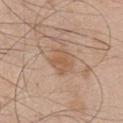Case summary:
* follow-up · no biopsy performed (imaged during a skin exam)
* patient · male, aged 43–47
* automated lesion analysis · a footprint of about 6.5 mm² and an eccentricity of roughly 0.6; a lesion color around L≈57 a*≈19 b*≈32 in CIELAB, a lesion–skin lightness drop of about 7, and a normalized border contrast of about 6; a color-variation rating of about 2/10 and peripheral color asymmetry of about 0.5; a classifier nevus-likeness of about 5/100 and a detector confidence of about 100 out of 100 that the crop contains a lesion
* imaging modality · ~15 mm crop, total-body skin-cancer survey
* size · about 3 mm
* tile lighting · white-light illumination
* body site · the chest